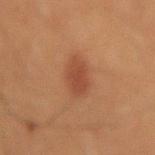Notes:
– follow-up — total-body-photography surveillance lesion; no biopsy
– location — the abdomen
– image source — ~15 mm crop, total-body skin-cancer survey
– patient — male, aged approximately 60
– lesion diameter — ≈3.5 mm